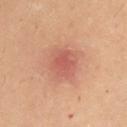workup = imaged on a skin check; not biopsied | lesion size = ≈3 mm | tile lighting = cross-polarized | site = the left upper arm | image-analysis metrics = an area of roughly 7.5 mm², a shape eccentricity near 0.4, and a symmetry-axis asymmetry near 0.15; a border-irregularity index near 1.5/10, a color-variation rating of about 2/10, and peripheral color asymmetry of about 0.5 | subject = male, roughly 30 years of age | imaging modality = ~15 mm crop, total-body skin-cancer survey.